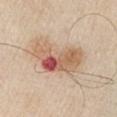| key | value |
|---|---|
| notes | imaged on a skin check; not biopsied |
| patient | male, roughly 80 years of age |
| body site | the left upper arm |
| illumination | white-light |
| acquisition | ~15 mm crop, total-body skin-cancer survey |